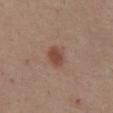biopsy status: catalogued during a skin exam; not biopsied | illumination: white-light | acquisition: ~15 mm crop, total-body skin-cancer survey | subject: male, aged around 55 | lesion diameter: ≈3 mm | site: the abdomen | automated lesion analysis: a footprint of about 4.5 mm², a shape eccentricity near 0.7, and a shape-asymmetry score of about 0.15 (0 = symmetric); an average lesion color of about L≈46 a*≈21 b*≈26 (CIELAB) and a lesion–skin lightness drop of about 10; an automated nevus-likeness rating near 95 out of 100 and lesion-presence confidence of about 100/100.